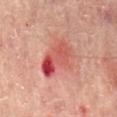Acquisition and patient details:
The lesion is located on the mid back. A close-up tile cropped from a whole-body skin photograph, about 15 mm across. A male patient in their mid-60s. Automated tile analysis of the lesion measured an area of roughly 14 mm² and a shape-asymmetry score of about 0.4 (0 = symmetric). The software also gave an average lesion color of about L≈54 a*≈34 b*≈28 (CIELAB), roughly 11 lightness units darker than nearby skin, and a lesion-to-skin contrast of about 7.5 (normalized; higher = more distinct). It also reported a border-irregularity index near 4/10, internal color variation of about 10 on a 0–10 scale, and peripheral color asymmetry of about 4. It also reported an automated nevus-likeness rating near 0 out of 100. The tile uses cross-polarized illumination.